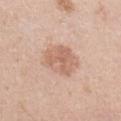Q: Was a biopsy performed?
A: catalogued during a skin exam; not biopsied
Q: What lighting was used for the tile?
A: white-light
Q: What is the imaging modality?
A: ~15 mm crop, total-body skin-cancer survey
Q: Automated lesion metrics?
A: an outline eccentricity of about 0.6 (0 = round, 1 = elongated) and a shape-asymmetry score of about 0.15 (0 = symmetric); an automated nevus-likeness rating near 10 out of 100
Q: What are the patient's age and sex?
A: male, approximately 45 years of age
Q: Where on the body is the lesion?
A: the right upper arm
Q: Lesion size?
A: ≈4.5 mm